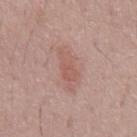{
  "biopsy_status": "not biopsied; imaged during a skin examination",
  "lesion_size": {
    "long_diameter_mm_approx": 5.0
  },
  "site": "chest",
  "patient": {
    "sex": "male",
    "age_approx": 45
  },
  "image": {
    "source": "total-body photography crop",
    "field_of_view_mm": 15
  },
  "lighting": "white-light",
  "automated_metrics": {
    "border_irregularity_0_10": 3.5,
    "color_variation_0_10": 2.0,
    "peripheral_color_asymmetry": 0.5,
    "lesion_detection_confidence_0_100": 100
  }
}